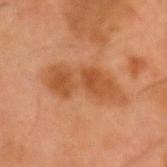Recorded during total-body skin imaging; not selected for excision or biopsy. The subject is a male approximately 55 years of age. Longest diameter approximately 8 mm. From the head or neck. Imaged with cross-polarized lighting. Cropped from a whole-body photographic skin survey; the tile spans about 15 mm.